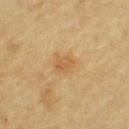Assessment:
This lesion was catalogued during total-body skin photography and was not selected for biopsy.
Context:
About 2.5 mm across. On the upper back. The subject is a female roughly 55 years of age. The tile uses cross-polarized illumination. A roughly 15 mm field-of-view crop from a total-body skin photograph.